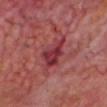| feature | finding |
|---|---|
| workup | total-body-photography surveillance lesion; no biopsy |
| patient | male, roughly 65 years of age |
| automated lesion analysis | a lesion color around L≈36 a*≈32 b*≈19 in CIELAB, about 10 CIELAB-L* units darker than the surrounding skin, and a normalized border contrast of about 8.5; a nevus-likeness score of about 0/100 |
| lesion size | ~5 mm (longest diameter) |
| image source | ~15 mm crop, total-body skin-cancer survey |
| lighting | cross-polarized |
| site | the head or neck |